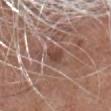Impression: Recorded during total-body skin imaging; not selected for excision or biopsy. Acquisition and patient details: Measured at roughly 2.5 mm in maximum diameter. A male patient approximately 75 years of age. The lesion is located on the head or neck. The tile uses white-light illumination. Automated image analysis of the tile measured a mean CIELAB color near L≈45 a*≈20 b*≈24, about 10 CIELAB-L* units darker than the surrounding skin, and a normalized lesion–skin contrast near 8. And it measured a border-irregularity index near 2.5/10, a within-lesion color-variation index near 3/10, and radial color variation of about 1. Cropped from a whole-body photographic skin survey; the tile spans about 15 mm.